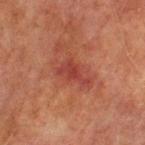Q: Was a biopsy performed?
A: catalogued during a skin exam; not biopsied
Q: Lesion location?
A: the left upper arm
Q: How was this image acquired?
A: total-body-photography crop, ~15 mm field of view
Q: Who is the patient?
A: male, roughly 75 years of age